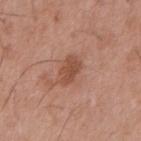Assessment: The lesion was tiled from a total-body skin photograph and was not biopsied. Clinical summary: A close-up tile cropped from a whole-body skin photograph, about 15 mm across. Approximately 3.5 mm at its widest. The patient is a male approximately 55 years of age. The lesion is on the front of the torso.